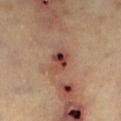| key | value |
|---|---|
| size | about 3 mm |
| body site | the left lower leg |
| automated metrics | an area of roughly 5.5 mm², an outline eccentricity of about 0.75 (0 = round, 1 = elongated), and a shape-asymmetry score of about 0.25 (0 = symmetric); a normalized lesion–skin contrast near 9.5; a border-irregularity rating of about 2.5/10, a within-lesion color-variation index near 9.5/10, and peripheral color asymmetry of about 5.5 |
| subject | approximately 60 years of age |
| imaging modality | ~15 mm tile from a whole-body skin photo |